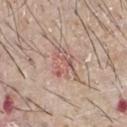Impression:
Captured during whole-body skin photography for melanoma surveillance; the lesion was not biopsied.
Clinical summary:
On the front of the torso. The patient is a male aged 63–67. A roughly 15 mm field-of-view crop from a total-body skin photograph. The lesion's longest dimension is about 4.5 mm. Imaged with white-light lighting.